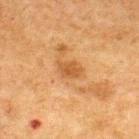Clinical impression: Captured during whole-body skin photography for melanoma surveillance; the lesion was not biopsied. Context: A male subject in their mid- to late 70s. Located on the upper back. A roughly 15 mm field-of-view crop from a total-body skin photograph.